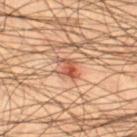Findings:
– notes: imaged on a skin check; not biopsied
– TBP lesion metrics: an area of roughly 4 mm², an eccentricity of roughly 0.85, and two-axis asymmetry of about 0.4; an average lesion color of about L≈51 a*≈26 b*≈32 (CIELAB), roughly 11 lightness units darker than nearby skin, and a normalized lesion–skin contrast near 8
– diameter: ~3.5 mm (longest diameter)
– illumination: cross-polarized illumination
– site: the right thigh
– acquisition: ~15 mm crop, total-body skin-cancer survey
– patient: male, aged around 55